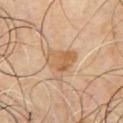biopsy_status: not biopsied; imaged during a skin examination